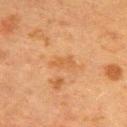| feature | finding |
|---|---|
| notes | no biopsy performed (imaged during a skin exam) |
| subject | female, aged around 55 |
| location | the upper back |
| lighting | cross-polarized illumination |
| image source | ~15 mm crop, total-body skin-cancer survey |
| lesion size | ≈3 mm |